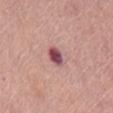This lesion was catalogued during total-body skin photography and was not selected for biopsy. A female patient, aged approximately 65. A 15 mm close-up tile from a total-body photography series done for melanoma screening. The lesion is on the abdomen.